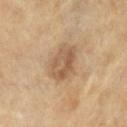Impression: The lesion was tiled from a total-body skin photograph and was not biopsied. Image and clinical context: On the chest. The patient is a male roughly 60 years of age. Imaged with cross-polarized lighting. A 15 mm close-up tile from a total-body photography series done for melanoma screening.